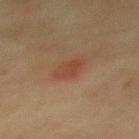<tbp_lesion>
<biopsy_status>not biopsied; imaged during a skin examination</biopsy_status>
<image>
  <source>total-body photography crop</source>
  <field_of_view_mm>15</field_of_view_mm>
</image>
<patient>
  <sex>female</sex>
  <age_approx>55</age_approx>
</patient>
<site>mid back</site>
</tbp_lesion>